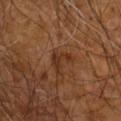Q: Is there a histopathology result?
A: total-body-photography surveillance lesion; no biopsy
Q: What is the anatomic site?
A: the right arm
Q: What kind of image is this?
A: total-body-photography crop, ~15 mm field of view
Q: Lesion size?
A: ~3 mm (longest diameter)
Q: How was the tile lit?
A: cross-polarized
Q: Patient demographics?
A: male, aged 58 to 62
Q: What did automated image analysis measure?
A: a footprint of about 4 mm² and a symmetry-axis asymmetry near 0.7; an average lesion color of about L≈31 a*≈20 b*≈30 (CIELAB), about 7 CIELAB-L* units darker than the surrounding skin, and a lesion-to-skin contrast of about 7 (normalized; higher = more distinct); a border-irregularity index near 8/10, a color-variation rating of about 0/10, and a peripheral color-asymmetry measure near 0